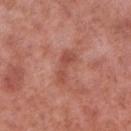This lesion was catalogued during total-body skin photography and was not selected for biopsy.
A 15 mm crop from a total-body photograph taken for skin-cancer surveillance.
The lesion is on the right lower leg.
A male subject aged 53 to 57.
Longest diameter approximately 3.5 mm.